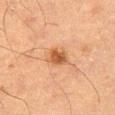automated lesion analysis=an area of roughly 6 mm², an eccentricity of roughly 0.65, and a symmetry-axis asymmetry near 0.2 | image source=15 mm crop, total-body photography | subject=male, aged 63–67 | anatomic site=the leg | lighting=cross-polarized illumination | size=about 3 mm.